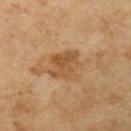Part of a total-body skin-imaging series; this lesion was reviewed on a skin check and was not flagged for biopsy.
A roughly 15 mm field-of-view crop from a total-body skin photograph.
An algorithmic analysis of the crop reported an area of roughly 11 mm² and an eccentricity of roughly 0.45. The software also gave an average lesion color of about L≈48 a*≈18 b*≈35 (CIELAB). And it measured border irregularity of about 4 on a 0–10 scale, a within-lesion color-variation index near 4/10, and peripheral color asymmetry of about 1.5.
Longest diameter approximately 4 mm.
The patient is a female roughly 60 years of age.
Captured under cross-polarized illumination.
On the left upper arm.